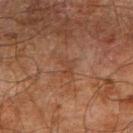follow-up = catalogued during a skin exam; not biopsied
size = ≈3 mm
patient = male, aged 68 to 72
anatomic site = the right upper arm
tile lighting = cross-polarized illumination
acquisition = ~15 mm crop, total-body skin-cancer survey
image-analysis metrics = a mean CIELAB color near L≈35 a*≈19 b*≈26, roughly 4 lightness units darker than nearby skin, and a normalized lesion–skin contrast near 4.5; a color-variation rating of about 0/10